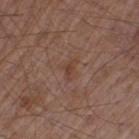{
  "biopsy_status": "not biopsied; imaged during a skin examination",
  "automated_metrics": {
    "area_mm2_approx": 3.0,
    "eccentricity": 0.85,
    "border_irregularity_0_10": 4.5,
    "color_variation_0_10": 1.0,
    "peripheral_color_asymmetry": 0.0
  },
  "lighting": "white-light",
  "lesion_size": {
    "long_diameter_mm_approx": 2.5
  },
  "image": {
    "source": "total-body photography crop",
    "field_of_view_mm": 15
  },
  "site": "left thigh",
  "patient": {
    "sex": "male",
    "age_approx": 55
  }
}